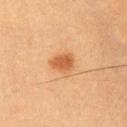Impression: The lesion was photographed on a routine skin check and not biopsied; there is no pathology result. Image and clinical context: Captured under cross-polarized illumination. The subject is a male aged 53–57. Automated image analysis of the tile measured an eccentricity of roughly 0.6. The software also gave a mean CIELAB color near L≈51 a*≈24 b*≈38 and roughly 11 lightness units darker than nearby skin. A 15 mm close-up extracted from a 3D total-body photography capture. Approximately 3 mm at its widest. On the left upper arm.